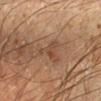patient: male, in their mid- to late 60s | automated lesion analysis: a footprint of about 5 mm², an outline eccentricity of about 0.85 (0 = round, 1 = elongated), and two-axis asymmetry of about 0.65; a mean CIELAB color near L≈39 a*≈16 b*≈27 and about 8 CIELAB-L* units darker than the surrounding skin | body site: the left forearm | lesion diameter: about 4 mm | image source: ~15 mm crop, total-body skin-cancer survey.